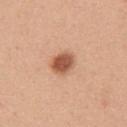Impression: Recorded during total-body skin imaging; not selected for excision or biopsy. Acquisition and patient details: The tile uses white-light illumination. The recorded lesion diameter is about 3.5 mm. The total-body-photography lesion software estimated a lesion area of about 6.5 mm², an outline eccentricity of about 0.65 (0 = round, 1 = elongated), and a symmetry-axis asymmetry near 0.15. It also reported an average lesion color of about L≈56 a*≈25 b*≈34 (CIELAB) and a lesion-to-skin contrast of about 9.5 (normalized; higher = more distinct). A female subject approximately 30 years of age. From the left upper arm. A lesion tile, about 15 mm wide, cut from a 3D total-body photograph.